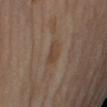Part of a total-body skin-imaging series; this lesion was reviewed on a skin check and was not flagged for biopsy. A female patient aged 63–67. The lesion is on the left arm. The lesion's longest dimension is about 3 mm. Captured under cross-polarized illumination. Cropped from a total-body skin-imaging series; the visible field is about 15 mm. The total-body-photography lesion software estimated an automated nevus-likeness rating near 0 out of 100 and a lesion-detection confidence of about 95/100.